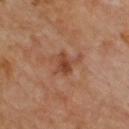subject = male, aged around 70
automated metrics = a footprint of about 4.5 mm²; a lesion–skin lightness drop of about 9; a border-irregularity index near 3/10, internal color variation of about 3.5 on a 0–10 scale, and radial color variation of about 1
image source = ~15 mm crop, total-body skin-cancer survey
anatomic site = the upper back
illumination = cross-polarized